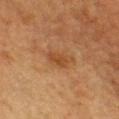This lesion was catalogued during total-body skin photography and was not selected for biopsy. A 15 mm close-up tile from a total-body photography series done for melanoma screening. About 3.5 mm across. The lesion-visualizer software estimated a mean CIELAB color near L≈38 a*≈20 b*≈32 and a lesion–skin lightness drop of about 7. It also reported an automated nevus-likeness rating near 25 out of 100. The tile uses cross-polarized illumination. Located on the head or neck. The subject is a female aged 53–57.